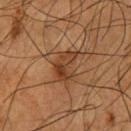Assessment: The lesion was photographed on a routine skin check and not biopsied; there is no pathology result. Background: On the front of the torso. A roughly 15 mm field-of-view crop from a total-body skin photograph. The tile uses cross-polarized illumination. The lesion's longest dimension is about 4 mm. A male subject, approximately 55 years of age.